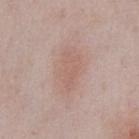Imaged during a routine full-body skin examination; the lesion was not biopsied and no histopathology is available. A male patient aged around 55. Cropped from a whole-body photographic skin survey; the tile spans about 15 mm. From the chest.